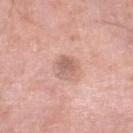Q: What is the anatomic site?
A: the right thigh
Q: What kind of image is this?
A: ~15 mm tile from a whole-body skin photo
Q: What did automated image analysis measure?
A: a shape eccentricity near 0.75 and a symmetry-axis asymmetry near 0.3; a mean CIELAB color near L≈60 a*≈21 b*≈26; a classifier nevus-likeness of about 0/100 and a lesion-detection confidence of about 100/100
Q: Patient demographics?
A: male, roughly 80 years of age